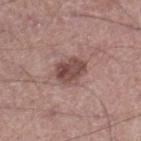Assessment:
No biopsy was performed on this lesion — it was imaged during a full skin examination and was not determined to be concerning.
Background:
About 3.5 mm across. The patient is a male aged 53 to 57. The lesion-visualizer software estimated a lesion-to-skin contrast of about 9 (normalized; higher = more distinct). It also reported a border-irregularity rating of about 2/10, a within-lesion color-variation index near 5/10, and radial color variation of about 1.5. The software also gave a nevus-likeness score of about 50/100 and a detector confidence of about 100 out of 100 that the crop contains a lesion. A 15 mm close-up tile from a total-body photography series done for melanoma screening. The lesion is on the right lower leg.